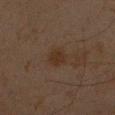The lesion is located on the right forearm. A close-up tile cropped from a whole-body skin photograph, about 15 mm across. The subject is a male in their mid- to late 40s. Measured at roughly 2.5 mm in maximum diameter. Automated image analysis of the tile measured a footprint of about 4.5 mm² and an outline eccentricity of about 0.4 (0 = round, 1 = elongated). It also reported a lesion color around L≈24 a*≈13 b*≈22 in CIELAB, about 5 CIELAB-L* units darker than the surrounding skin, and a normalized lesion–skin contrast near 7. And it measured a classifier nevus-likeness of about 25/100. Captured under cross-polarized illumination.